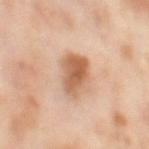Case summary:
• workup: total-body-photography surveillance lesion; no biopsy
• location: the right thigh
• image: ~15 mm tile from a whole-body skin photo
• tile lighting: cross-polarized
• patient: female, aged around 50
• lesion diameter: ≈4.5 mm